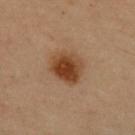The lesion was tiled from a total-body skin photograph and was not biopsied.
The lesion is located on the front of the torso.
This image is a 15 mm lesion crop taken from a total-body photograph.
The tile uses cross-polarized illumination.
A female patient, aged approximately 50.
An algorithmic analysis of the crop reported a footprint of about 12 mm², an eccentricity of roughly 0.45, and a shape-asymmetry score of about 0.15 (0 = symmetric). It also reported a lesion color around L≈36 a*≈18 b*≈30 in CIELAB, a lesion–skin lightness drop of about 11, and a lesion-to-skin contrast of about 10 (normalized; higher = more distinct).
About 4 mm across.